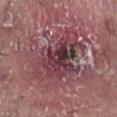illumination = white-light illumination | diameter = ≈8.5 mm | acquisition = ~15 mm tile from a whole-body skin photo | patient = female, aged 73 to 77 | anatomic site = the right lower leg.